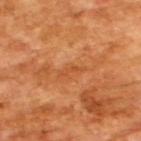<record>
<biopsy_status>not biopsied; imaged during a skin examination</biopsy_status>
<automated_metrics>
  <cielab_L>51</cielab_L>
  <cielab_a>29</cielab_a>
  <cielab_b>43</cielab_b>
  <vs_skin_darker_L>6.0</vs_skin_darker_L>
  <vs_skin_contrast_norm>5.0</vs_skin_contrast_norm>
  <border_irregularity_0_10>5.5</border_irregularity_0_10>
  <peripheral_color_asymmetry>0.0</peripheral_color_asymmetry>
</automated_metrics>
<lighting>cross-polarized</lighting>
<patient>
  <sex>male</sex>
  <age_approx>65</age_approx>
</patient>
<lesion_size>
  <long_diameter_mm_approx>3.0</long_diameter_mm_approx>
</lesion_size>
<image>
  <source>total-body photography crop</source>
  <field_of_view_mm>15</field_of_view_mm>
</image>
</record>